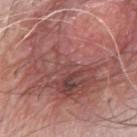Clinical impression: Part of a total-body skin-imaging series; this lesion was reviewed on a skin check and was not flagged for biopsy.